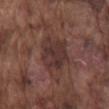This lesion was catalogued during total-body skin photography and was not selected for biopsy. The lesion is located on the mid back. Captured under white-light illumination. Longest diameter approximately 5.5 mm. The subject is a male roughly 75 years of age. A 15 mm close-up extracted from a 3D total-body photography capture.